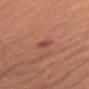  biopsy_status: not biopsied; imaged during a skin examination
  lighting: white-light
  patient:
    sex: female
    age_approx: 55
  lesion_size:
    long_diameter_mm_approx: 2.5
  site: arm
  image:
    source: total-body photography crop
    field_of_view_mm: 15